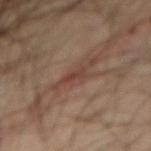Captured during whole-body skin photography for melanoma surveillance; the lesion was not biopsied.
Captured under cross-polarized illumination.
A male subject aged 38–42.
The lesion's longest dimension is about 2.5 mm.
From the right forearm.
Cropped from a whole-body photographic skin survey; the tile spans about 15 mm.